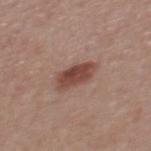biopsy_status: not biopsied; imaged during a skin examination
lighting: white-light
lesion_size:
  long_diameter_mm_approx: 4.0
image:
  source: total-body photography crop
  field_of_view_mm: 15
patient:
  sex: male
  age_approx: 55
site: mid back
automated_metrics:
  area_mm2_approx: 7.5
  eccentricity: 0.8
  shape_asymmetry: 0.2
  border_irregularity_0_10: 2.5